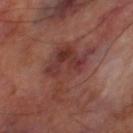Case summary:
– notes — catalogued during a skin exam; not biopsied
– tile lighting — cross-polarized
– subject — male, about 70 years old
– diameter — ≈7.5 mm
– image source — ~15 mm crop, total-body skin-cancer survey
– site — the left thigh